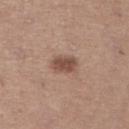Recorded during total-body skin imaging; not selected for excision or biopsy.
A female subject, roughly 45 years of age.
From the right lower leg.
The total-body-photography lesion software estimated a shape eccentricity near 0.75 and two-axis asymmetry of about 0.2. The software also gave about 12 CIELAB-L* units darker than the surrounding skin and a normalized border contrast of about 8.5. The software also gave a classifier nevus-likeness of about 85/100 and a detector confidence of about 100 out of 100 that the crop contains a lesion.
Longest diameter approximately 3 mm.
A close-up tile cropped from a whole-body skin photograph, about 15 mm across.
Captured under white-light illumination.